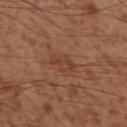Impression: Imaged during a routine full-body skin examination; the lesion was not biopsied and no histopathology is available. Context: Imaged with cross-polarized lighting. A male subject, aged around 55. On the left thigh. The total-body-photography lesion software estimated an area of roughly 4 mm², an eccentricity of roughly 0.85, and a symmetry-axis asymmetry near 0.25. The analysis additionally found an average lesion color of about L≈40 a*≈23 b*≈29 (CIELAB), a lesion–skin lightness drop of about 6, and a lesion-to-skin contrast of about 5 (normalized; higher = more distinct). The software also gave border irregularity of about 3.5 on a 0–10 scale, a color-variation rating of about 1.5/10, and peripheral color asymmetry of about 0.5. The analysis additionally found a nevus-likeness score of about 0/100 and lesion-presence confidence of about 100/100. A region of skin cropped from a whole-body photographic capture, roughly 15 mm wide. The recorded lesion diameter is about 3 mm.